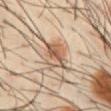Q: Is there a histopathology result?
A: total-body-photography surveillance lesion; no biopsy
Q: What are the patient's age and sex?
A: male, aged approximately 40
Q: Where on the body is the lesion?
A: the abdomen
Q: What kind of image is this?
A: ~15 mm tile from a whole-body skin photo
Q: Illumination type?
A: cross-polarized
Q: Lesion size?
A: about 3.5 mm